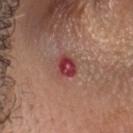Clinical impression: No biopsy was performed on this lesion — it was imaged during a full skin examination and was not determined to be concerning. Image and clinical context: From the head or neck. The recorded lesion diameter is about 3 mm. Cropped from a whole-body photographic skin survey; the tile spans about 15 mm. Captured under white-light illumination. The lesion-visualizer software estimated a lesion area of about 5 mm², a shape eccentricity near 0.55, and a symmetry-axis asymmetry near 0.25. And it measured a nevus-likeness score of about 0/100 and lesion-presence confidence of about 100/100. The patient is a male aged approximately 40.